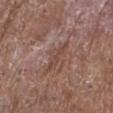The lesion was tiled from a total-body skin photograph and was not biopsied. A female patient roughly 80 years of age. Cropped from a whole-body photographic skin survey; the tile spans about 15 mm. The lesion is on the right lower leg.